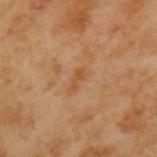| field | value |
|---|---|
| biopsy status | catalogued during a skin exam; not biopsied |
| acquisition | ~15 mm crop, total-body skin-cancer survey |
| automated lesion analysis | a lesion area of about 2 mm² and a shape eccentricity near 0.9; an average lesion color of about L≈50 a*≈22 b*≈38 (CIELAB), a lesion–skin lightness drop of about 6, and a normalized border contrast of about 5.5; border irregularity of about 5 on a 0–10 scale, a color-variation rating of about 0/10, and peripheral color asymmetry of about 0 |
| lighting | cross-polarized |
| subject | male, about 65 years old |
| lesion diameter | ≈2.5 mm |